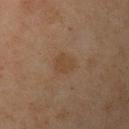Q: Was this lesion biopsied?
A: catalogued during a skin exam; not biopsied
Q: Illumination type?
A: cross-polarized illumination
Q: What kind of image is this?
A: total-body-photography crop, ~15 mm field of view
Q: Patient demographics?
A: female, aged 43 to 47
Q: Where on the body is the lesion?
A: the arm